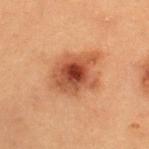  biopsy_status: not biopsied; imaged during a skin examination
  site: upper back
  image:
    source: total-body photography crop
    field_of_view_mm: 15
  patient:
    sex: female
    age_approx: 20
  lesion_size:
    long_diameter_mm_approx: 6.0
  automated_metrics:
    lesion_detection_confidence_0_100: 100
  lighting: cross-polarized